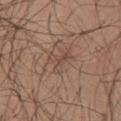Notes:
- follow-up: total-body-photography surveillance lesion; no biopsy
- patient: male, aged around 25
- anatomic site: the upper back
- image: total-body-photography crop, ~15 mm field of view